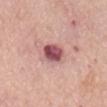Assessment: Recorded during total-body skin imaging; not selected for excision or biopsy. Image and clinical context: From the mid back. The tile uses white-light illumination. A male patient in their mid- to late 70s. This image is a 15 mm lesion crop taken from a total-body photograph.